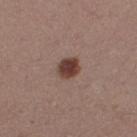follow-up: imaged on a skin check; not biopsied | location: the right thigh | automated lesion analysis: a footprint of about 5.5 mm², an outline eccentricity of about 0.65 (0 = round, 1 = elongated), and a symmetry-axis asymmetry near 0.2; a mean CIELAB color near L≈40 a*≈19 b*≈23 and about 14 CIELAB-L* units darker than the surrounding skin; a border-irregularity index near 1.5/10, a within-lesion color-variation index near 3/10, and a peripheral color-asymmetry measure near 1; a nevus-likeness score of about 100/100 and lesion-presence confidence of about 100/100 | lesion diameter: ≈3 mm | illumination: white-light illumination | acquisition: total-body-photography crop, ~15 mm field of view | patient: female, aged around 30.